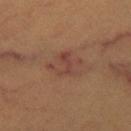notes=total-body-photography surveillance lesion; no biopsy | TBP lesion metrics=a within-lesion color-variation index near 5/10 and peripheral color asymmetry of about 2; a lesion-detection confidence of about 100/100 | tile lighting=cross-polarized | image source=~15 mm crop, total-body skin-cancer survey | subject=female, aged around 45 | anatomic site=the leg | size=~4.5 mm (longest diameter).